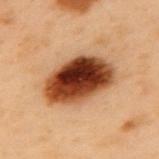Findings:
- follow-up · imaged on a skin check; not biopsied
- image source · ~15 mm crop, total-body skin-cancer survey
- patient · male, aged 53 to 57
- illumination · cross-polarized
- anatomic site · the mid back